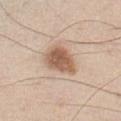Clinical impression: Captured during whole-body skin photography for melanoma surveillance; the lesion was not biopsied. Background: The recorded lesion diameter is about 4.5 mm. A male patient approximately 45 years of age. This image is a 15 mm lesion crop taken from a total-body photograph. From the chest.